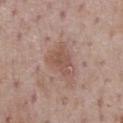biopsy status: imaged on a skin check; not biopsied | lighting: white-light illumination | imaging modality: total-body-photography crop, ~15 mm field of view | lesion size: ≈4 mm | patient: male, aged 73–77 | location: the chest.